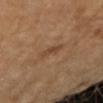Recorded during total-body skin imaging; not selected for excision or biopsy. The subject is roughly 60 years of age. A lesion tile, about 15 mm wide, cut from a 3D total-body photograph. Approximately 3 mm at its widest. The lesion is on the right forearm. The lesion-visualizer software estimated an area of roughly 4 mm² and a shape-asymmetry score of about 0.25 (0 = symmetric). It also reported a lesion color around L≈44 a*≈18 b*≈32 in CIELAB. The tile uses cross-polarized illumination.